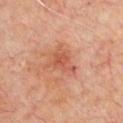Clinical impression: This lesion was catalogued during total-body skin photography and was not selected for biopsy. Context: A region of skin cropped from a whole-body photographic capture, roughly 15 mm wide. The lesion is on the chest. A male patient in their 60s. Longest diameter approximately 4 mm. Captured under cross-polarized illumination. The total-body-photography lesion software estimated a footprint of about 8 mm², an outline eccentricity of about 0.7 (0 = round, 1 = elongated), and two-axis asymmetry of about 0.4. It also reported a nevus-likeness score of about 5/100 and a detector confidence of about 100 out of 100 that the crop contains a lesion.